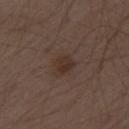Q: Was this lesion biopsied?
A: no biopsy performed (imaged during a skin exam)
Q: Automated lesion metrics?
A: an average lesion color of about L≈31 a*≈15 b*≈22 (CIELAB), roughly 6 lightness units darker than nearby skin, and a normalized border contrast of about 7
Q: Illumination type?
A: white-light illumination
Q: Lesion location?
A: the left thigh
Q: How was this image acquired?
A: ~15 mm tile from a whole-body skin photo
Q: What are the patient's age and sex?
A: male, approximately 70 years of age